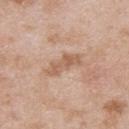Acquisition and patient details: About 4.5 mm across. Imaged with white-light lighting. A lesion tile, about 15 mm wide, cut from a 3D total-body photograph. The lesion is located on the back. A male patient, aged 23 to 27.